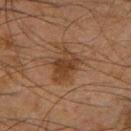Clinical impression:
The lesion was tiled from a total-body skin photograph and was not biopsied.
Clinical summary:
A 15 mm crop from a total-body photograph taken for skin-cancer surveillance. The recorded lesion diameter is about 4 mm. Captured under cross-polarized illumination. The total-body-photography lesion software estimated an area of roughly 8.5 mm², an outline eccentricity of about 0.75 (0 = round, 1 = elongated), and two-axis asymmetry of about 0.35. And it measured a border-irregularity rating of about 4/10. A male subject, aged 63 to 67. On the left thigh.